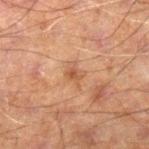Q: Was this lesion biopsied?
A: no biopsy performed (imaged during a skin exam)
Q: What is the anatomic site?
A: the leg
Q: What did automated image analysis measure?
A: an average lesion color of about L≈42 a*≈18 b*≈28 (CIELAB), a lesion–skin lightness drop of about 7, and a normalized lesion–skin contrast near 6; a border-irregularity rating of about 4/10, internal color variation of about 2.5 on a 0–10 scale, and radial color variation of about 1; a classifier nevus-likeness of about 0/100 and a lesion-detection confidence of about 100/100
Q: What lighting was used for the tile?
A: cross-polarized
Q: Who is the patient?
A: male, aged approximately 60
Q: What kind of image is this?
A: ~15 mm tile from a whole-body skin photo
Q: What is the lesion's diameter?
A: about 2.5 mm